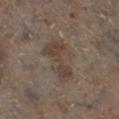Notes:
• notes · total-body-photography surveillance lesion; no biopsy
• TBP lesion metrics · an area of roughly 11 mm², an outline eccentricity of about 0.9 (0 = round, 1 = elongated), and two-axis asymmetry of about 0.4; about 7 CIELAB-L* units darker than the surrounding skin and a normalized border contrast of about 6.5; a border-irregularity rating of about 6/10 and radial color variation of about 1; a classifier nevus-likeness of about 5/100
• tile lighting · cross-polarized illumination
• location · the left lower leg
• patient · female, about 60 years old
• acquisition · ~15 mm tile from a whole-body skin photo
• lesion diameter · ~5.5 mm (longest diameter)